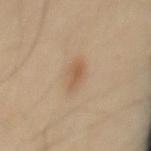Findings:
• subject · male, aged approximately 45
• acquisition · total-body-photography crop, ~15 mm field of view
• anatomic site · the mid back
• illumination · cross-polarized illumination
• size · ≈3.5 mm
• TBP lesion metrics · a shape eccentricity near 0.85; a mean CIELAB color near L≈55 a*≈17 b*≈32; a border-irregularity rating of about 3/10, internal color variation of about 2.5 on a 0–10 scale, and radial color variation of about 1; a nevus-likeness score of about 80/100 and lesion-presence confidence of about 100/100